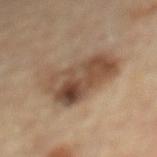The tile uses cross-polarized illumination. A male subject, aged around 85 or older. From the back. The lesion-visualizer software estimated a footprint of about 26 mm², a shape eccentricity near 0.8, and two-axis asymmetry of about 0.4. The software also gave a lesion color around L≈46 a*≈15 b*≈28 in CIELAB, about 12 CIELAB-L* units darker than the surrounding skin, and a normalized lesion–skin contrast near 9. Cropped from a total-body skin-imaging series; the visible field is about 15 mm.